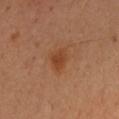Assessment:
No biopsy was performed on this lesion — it was imaged during a full skin examination and was not determined to be concerning.
Acquisition and patient details:
On the left forearm. The tile uses cross-polarized illumination. The subject is a female approximately 50 years of age. A 15 mm close-up extracted from a 3D total-body photography capture.